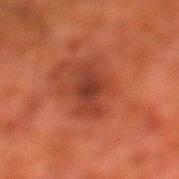Q: Was this lesion biopsied?
A: no biopsy performed (imaged during a skin exam)
Q: What is the lesion's diameter?
A: about 5 mm
Q: What did automated image analysis measure?
A: a footprint of about 9 mm² and an outline eccentricity of about 0.9 (0 = round, 1 = elongated); an average lesion color of about L≈31 a*≈25 b*≈27 (CIELAB), roughly 8 lightness units darker than nearby skin, and a lesion-to-skin contrast of about 7.5 (normalized; higher = more distinct)
Q: Where on the body is the lesion?
A: the left lower leg
Q: Who is the patient?
A: male, roughly 80 years of age
Q: What is the imaging modality?
A: total-body-photography crop, ~15 mm field of view